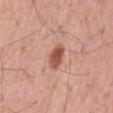The lesion was photographed on a routine skin check and not biopsied; there is no pathology result.
An algorithmic analysis of the crop reported a lesion area of about 5 mm², a shape eccentricity near 0.75, and a shape-asymmetry score of about 0.2 (0 = symmetric).
Imaged with white-light lighting.
A 15 mm close-up tile from a total-body photography series done for melanoma screening.
Approximately 3 mm at its widest.
A male subject, in their mid- to late 50s.
The lesion is located on the mid back.